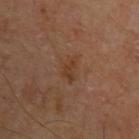Impression:
The lesion was photographed on a routine skin check and not biopsied; there is no pathology result.
Image and clinical context:
A male subject, aged approximately 70. Imaged with cross-polarized lighting. A 15 mm crop from a total-body photograph taken for skin-cancer surveillance. Located on the left upper arm.